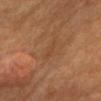Notes:
• biopsy status · total-body-photography surveillance lesion; no biopsy
• TBP lesion metrics · an average lesion color of about L≈43 a*≈21 b*≈32 (CIELAB) and a lesion–skin lightness drop of about 4; border irregularity of about 3 on a 0–10 scale, a within-lesion color-variation index near 0.5/10, and radial color variation of about 0
• site · the head or neck
• patient · female, about 65 years old
• tile lighting · cross-polarized
• acquisition · 15 mm crop, total-body photography